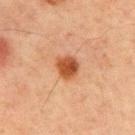Clinical impression: Captured during whole-body skin photography for melanoma surveillance; the lesion was not biopsied. Clinical summary: Captured under cross-polarized illumination. This image is a 15 mm lesion crop taken from a total-body photograph. A male subject, in their mid- to late 60s. Longest diameter approximately 2.5 mm. Automated image analysis of the tile measured a mean CIELAB color near L≈41 a*≈24 b*≈33 and a normalized border contrast of about 10.5. The analysis additionally found border irregularity of about 1.5 on a 0–10 scale and radial color variation of about 1. It also reported a classifier nevus-likeness of about 100/100 and a lesion-detection confidence of about 100/100. Located on the chest.